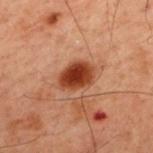No biopsy was performed on this lesion — it was imaged during a full skin examination and was not determined to be concerning.